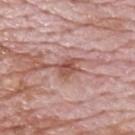The lesion was photographed on a routine skin check and not biopsied; there is no pathology result.
On the upper back.
A region of skin cropped from a whole-body photographic capture, roughly 15 mm wide.
The subject is a male in their 70s.
Measured at roughly 3 mm in maximum diameter.
Imaged with white-light lighting.